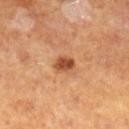{"lesion_size": {"long_diameter_mm_approx": 2.5}, "site": "right lower leg", "lighting": "cross-polarized", "image": {"source": "total-body photography crop", "field_of_view_mm": 15}, "automated_metrics": {"area_mm2_approx": 4.0, "eccentricity": 0.7, "shape_asymmetry": 0.2, "cielab_L": 46, "cielab_a": 24, "cielab_b": 34, "vs_skin_darker_L": 13.0, "vs_skin_contrast_norm": 9.5, "nevus_likeness_0_100": 90, "lesion_detection_confidence_0_100": 100}, "patient": {"sex": "male", "age_approx": 60}}